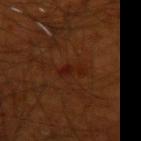No biopsy was performed on this lesion — it was imaged during a full skin examination and was not determined to be concerning.
The lesion is located on the left forearm.
A lesion tile, about 15 mm wide, cut from a 3D total-body photograph.
The subject is a male approximately 70 years of age.